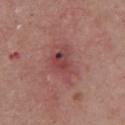Assessment:
This lesion was catalogued during total-body skin photography and was not selected for biopsy.
Image and clinical context:
Approximately 5.5 mm at its widest. The patient is a male aged 58 to 62. The lesion is located on the chest. This is a white-light tile. The total-body-photography lesion software estimated a lesion area of about 13 mm², a shape eccentricity near 0.8, and a symmetry-axis asymmetry near 0.3. And it measured a lesion color around L≈46 a*≈26 b*≈22 in CIELAB and a lesion–skin lightness drop of about 8. And it measured a border-irregularity rating of about 4/10 and peripheral color asymmetry of about 2.5. A 15 mm crop from a total-body photograph taken for skin-cancer surveillance.